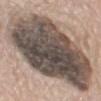<record>
<site>mid back</site>
<patient>
  <sex>male</sex>
  <age_approx>75</age_approx>
</patient>
<lesion_size>
  <long_diameter_mm_approx>14.0</long_diameter_mm_approx>
</lesion_size>
<automated_metrics>
  <border_irregularity_0_10>2.5</border_irregularity_0_10>
  <color_variation_0_10>9.5</color_variation_0_10>
  <peripheral_color_asymmetry>3.0</peripheral_color_asymmetry>
  <lesion_detection_confidence_0_100>35</lesion_detection_confidence_0_100>
</automated_metrics>
<image>
  <source>total-body photography crop</source>
  <field_of_view_mm>15</field_of_view_mm>
</image>
</record>